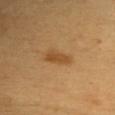notes = imaged on a skin check; not biopsied | acquisition = 15 mm crop, total-body photography | lighting = cross-polarized illumination | lesion diameter = ≈3.5 mm | site = the chest | subject = female, aged around 50.